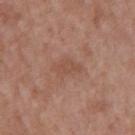Clinical impression:
This lesion was catalogued during total-body skin photography and was not selected for biopsy.
Image and clinical context:
The lesion is on the mid back. This is a white-light tile. Longest diameter approximately 3.5 mm. Cropped from a total-body skin-imaging series; the visible field is about 15 mm. A male subject aged 48–52.